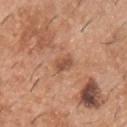The lesion was photographed on a routine skin check and not biopsied; there is no pathology result. The lesion is on the chest. This is a white-light tile. The subject is a male aged around 40. This image is a 15 mm lesion crop taken from a total-body photograph. Automated tile analysis of the lesion measured a nevus-likeness score of about 15/100 and a lesion-detection confidence of about 100/100. Longest diameter approximately 2.5 mm.